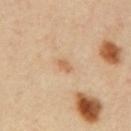Part of a total-body skin-imaging series; this lesion was reviewed on a skin check and was not flagged for biopsy. A 15 mm close-up extracted from a 3D total-body photography capture. Approximately 2 mm at its widest. Automated tile analysis of the lesion measured a lesion area of about 1.5 mm². The analysis additionally found a mean CIELAB color near L≈63 a*≈19 b*≈37, about 9 CIELAB-L* units darker than the surrounding skin, and a normalized lesion–skin contrast near 6.5. The analysis additionally found an automated nevus-likeness rating near 10 out of 100. The subject is a male about 40 years old. The tile uses cross-polarized illumination. From the mid back.